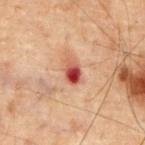Q: Was a biopsy performed?
A: catalogued during a skin exam; not biopsied
Q: What is the imaging modality?
A: total-body-photography crop, ~15 mm field of view
Q: Illumination type?
A: cross-polarized illumination
Q: Lesion location?
A: the chest
Q: Who is the patient?
A: male, about 70 years old
Q: What did automated image analysis measure?
A: a mean CIELAB color near L≈39 a*≈26 b*≈25, about 14 CIELAB-L* units darker than the surrounding skin, and a normalized lesion–skin contrast near 11; a classifier nevus-likeness of about 0/100